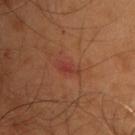This lesion was catalogued during total-body skin photography and was not selected for biopsy.
The lesion is on the back.
Cropped from a whole-body photographic skin survey; the tile spans about 15 mm.
A male patient roughly 55 years of age.